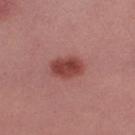Captured during whole-body skin photography for melanoma surveillance; the lesion was not biopsied.
The recorded lesion diameter is about 3.5 mm.
A 15 mm close-up tile from a total-body photography series done for melanoma screening.
The patient is a male about 40 years old.
The lesion is located on the right upper arm.